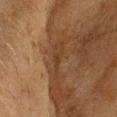workup — catalogued during a skin exam; not biopsied
location — the head or neck
acquisition — ~15 mm tile from a whole-body skin photo
diameter — about 2.5 mm
subject — male, about 60 years old
automated lesion analysis — an area of roughly 2 mm², an eccentricity of roughly 0.9, and a shape-asymmetry score of about 0.5 (0 = symmetric); roughly 5 lightness units darker than nearby skin and a normalized border contrast of about 5; a nevus-likeness score of about 0/100 and a lesion-detection confidence of about 65/100
illumination — cross-polarized